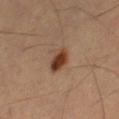{"patient": {"sex": "female", "age_approx": 55}, "lighting": "cross-polarized", "site": "leg", "image": {"source": "total-body photography crop", "field_of_view_mm": 15}}